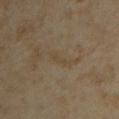workup: imaged on a skin check; not biopsied | body site: the upper back | patient: female, in their mid-30s | acquisition: 15 mm crop, total-body photography.